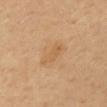Assessment:
This lesion was catalogued during total-body skin photography and was not selected for biopsy.
Acquisition and patient details:
Captured under cross-polarized illumination. On the back. An algorithmic analysis of the crop reported an outline eccentricity of about 0.85 (0 = round, 1 = elongated) and two-axis asymmetry of about 0.3. Approximately 3.5 mm at its widest. A male subject, roughly 40 years of age. A region of skin cropped from a whole-body photographic capture, roughly 15 mm wide.